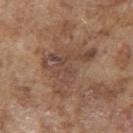Recorded during total-body skin imaging; not selected for excision or biopsy.